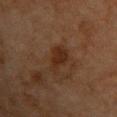Impression: Captured during whole-body skin photography for melanoma surveillance; the lesion was not biopsied. Clinical summary: The lesion is located on the upper back. Longest diameter approximately 3.5 mm. The total-body-photography lesion software estimated a lesion area of about 6.5 mm². And it measured a lesion color around L≈24 a*≈17 b*≈25 in CIELAB and a normalized border contrast of about 8. The software also gave a classifier nevus-likeness of about 55/100 and lesion-presence confidence of about 100/100. This image is a 15 mm lesion crop taken from a total-body photograph. A female patient, approximately 70 years of age. The tile uses cross-polarized illumination.